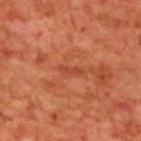{"biopsy_status": "not biopsied; imaged during a skin examination", "lesion_size": {"long_diameter_mm_approx": 2.5}, "lighting": "cross-polarized", "patient": {"sex": "male", "age_approx": 70}, "image": {"source": "total-body photography crop", "field_of_view_mm": 15}, "site": "back", "automated_metrics": {"area_mm2_approx": 1.5, "eccentricity": 0.95, "shape_asymmetry": 0.35, "cielab_L": 45, "cielab_a": 34, "cielab_b": 36, "vs_skin_darker_L": 7.0, "vs_skin_contrast_norm": 5.0, "color_variation_0_10": 0.0, "peripheral_color_asymmetry": 0.0, "nevus_likeness_0_100": 0, "lesion_detection_confidence_0_100": 95}}